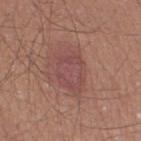The lesion was tiled from a total-body skin photograph and was not biopsied. The lesion is on the right thigh. A close-up tile cropped from a whole-body skin photograph, about 15 mm across. Captured under white-light illumination. Longest diameter approximately 5 mm. A male patient about 35 years old.